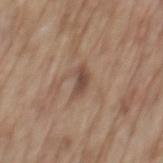{"patient": {"sex": "male", "age_approx": 70}, "image": {"source": "total-body photography crop", "field_of_view_mm": 15}, "site": "mid back"}